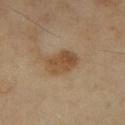{
  "biopsy_status": "not biopsied; imaged during a skin examination",
  "site": "right lower leg",
  "lighting": "cross-polarized",
  "automated_metrics": {
    "area_mm2_approx": 8.5,
    "eccentricity": 0.75,
    "color_variation_0_10": 4.0,
    "peripheral_color_asymmetry": 1.5
  },
  "patient": {
    "sex": "female",
    "age_approx": 70
  },
  "image": {
    "source": "total-body photography crop",
    "field_of_view_mm": 15
  }
}